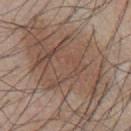This lesion was catalogued during total-body skin photography and was not selected for biopsy.
The patient is a male aged 53 to 57.
Located on the front of the torso.
Longest diameter approximately 14 mm.
A roughly 15 mm field-of-view crop from a total-body skin photograph.